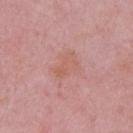biopsy_status: not biopsied; imaged during a skin examination
image:
  source: total-body photography crop
  field_of_view_mm: 15
site: chest
patient:
  sex: male
  age_approx: 50